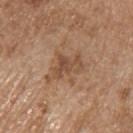Assessment:
Imaged during a routine full-body skin examination; the lesion was not biopsied and no histopathology is available.
Clinical summary:
Located on the chest. A lesion tile, about 15 mm wide, cut from a 3D total-body photograph. A male subject, aged around 70.